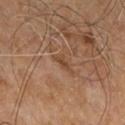notes: imaged on a skin check; not biopsied | image-analysis metrics: a shape eccentricity near 0.85 and two-axis asymmetry of about 0.4; a border-irregularity index near 4/10, internal color variation of about 0 on a 0–10 scale, and radial color variation of about 0; a nevus-likeness score of about 0/100 and a detector confidence of about 95 out of 100 that the crop contains a lesion | imaging modality: ~15 mm tile from a whole-body skin photo | subject: male, aged approximately 60 | lighting: cross-polarized illumination | location: the lower back.